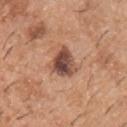This lesion was catalogued during total-body skin photography and was not selected for biopsy. A male patient in their 40s. Imaged with white-light lighting. A lesion tile, about 15 mm wide, cut from a 3D total-body photograph. The total-body-photography lesion software estimated a shape eccentricity near 0.55 and a symmetry-axis asymmetry near 0.3. The software also gave an average lesion color of about L≈47 a*≈21 b*≈27 (CIELAB) and a normalized border contrast of about 11.5. The analysis additionally found a border-irregularity index near 3/10, internal color variation of about 7 on a 0–10 scale, and a peripheral color-asymmetry measure near 2.5. The analysis additionally found a classifier nevus-likeness of about 0/100 and a lesion-detection confidence of about 100/100. From the front of the torso.